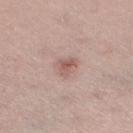biopsy status = catalogued during a skin exam; not biopsied | body site = the right thigh | tile lighting = white-light illumination | diameter = ≈2.5 mm | TBP lesion metrics = a footprint of about 5 mm² and a symmetry-axis asymmetry near 0.3; a nevus-likeness score of about 60/100 and a lesion-detection confidence of about 100/100 | image = ~15 mm crop, total-body skin-cancer survey | subject = female, aged 33–37.